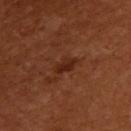workup = no biopsy performed (imaged during a skin exam)
image = total-body-photography crop, ~15 mm field of view
illumination = cross-polarized
location = the upper back
lesion size = ≈3 mm
patient = female, aged 53–57